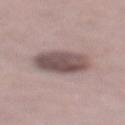Q: Is there a histopathology result?
A: catalogued during a skin exam; not biopsied
Q: What did automated image analysis measure?
A: an eccentricity of roughly 0.55 and a shape-asymmetry score of about 0.15 (0 = symmetric); a mean CIELAB color near L≈51 a*≈16 b*≈17 and about 14 CIELAB-L* units darker than the surrounding skin; a color-variation rating of about 5/10 and a peripheral color-asymmetry measure near 1.5
Q: Who is the patient?
A: female, roughly 65 years of age
Q: How was the tile lit?
A: white-light illumination
Q: What is the lesion's diameter?
A: ~5 mm (longest diameter)
Q: What is the anatomic site?
A: the left lower leg
Q: How was this image acquired?
A: total-body-photography crop, ~15 mm field of view